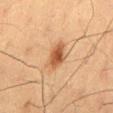Q: Is there a histopathology result?
A: total-body-photography surveillance lesion; no biopsy
Q: What is the imaging modality?
A: ~15 mm crop, total-body skin-cancer survey
Q: What is the anatomic site?
A: the mid back
Q: Lesion size?
A: ≈4 mm
Q: What lighting was used for the tile?
A: cross-polarized illumination
Q: What are the patient's age and sex?
A: male, aged 58 to 62
Q: What did automated image analysis measure?
A: a shape eccentricity near 0.75 and two-axis asymmetry of about 0.2